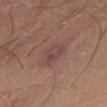workup = total-body-photography surveillance lesion; no biopsy
subject = female, approximately 35 years of age
anatomic site = the front of the torso
lesion diameter = about 3 mm
TBP lesion metrics = a mean CIELAB color near L≈44 a*≈19 b*≈21, a lesion–skin lightness drop of about 6, and a normalized lesion–skin contrast near 6; a border-irregularity index near 2.5/10, internal color variation of about 3.5 on a 0–10 scale, and a peripheral color-asymmetry measure near 1.5
illumination = white-light
image source = 15 mm crop, total-body photography